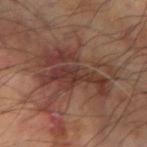This lesion was catalogued during total-body skin photography and was not selected for biopsy.
Automated tile analysis of the lesion measured an average lesion color of about L≈36 a*≈19 b*≈23 (CIELAB), a lesion–skin lightness drop of about 8, and a normalized border contrast of about 8.
On the left arm.
A male patient, roughly 60 years of age.
A 15 mm close-up extracted from a 3D total-body photography capture.
Longest diameter approximately 9 mm.
The tile uses cross-polarized illumination.